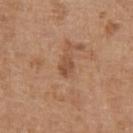Assessment:
The lesion was tiled from a total-body skin photograph and was not biopsied.
Background:
The lesion is located on the chest. Approximately 2.5 mm at its widest. A lesion tile, about 15 mm wide, cut from a 3D total-body photograph. This is a white-light tile. An algorithmic analysis of the crop reported an area of roughly 3.5 mm² and an outline eccentricity of about 0.8 (0 = round, 1 = elongated). The patient is a male in their 70s.